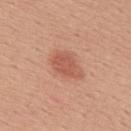Case summary:
• biopsy status — imaged on a skin check; not biopsied
• size — ≈3.5 mm
• imaging modality — total-body-photography crop, ~15 mm field of view
• patient — male, about 55 years old
• body site — the upper back